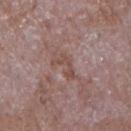Imaged during a routine full-body skin examination; the lesion was not biopsied and no histopathology is available.
A region of skin cropped from a whole-body photographic capture, roughly 15 mm wide.
A male subject about 65 years old.
From the chest.